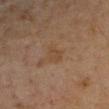Part of a total-body skin-imaging series; this lesion was reviewed on a skin check and was not flagged for biopsy. The patient is a female approximately 65 years of age. Cropped from a whole-body photographic skin survey; the tile spans about 15 mm. Automated image analysis of the tile measured an area of roughly 3.5 mm² and a shape-asymmetry score of about 0.45 (0 = symmetric). The analysis additionally found a classifier nevus-likeness of about 0/100. From the left forearm.